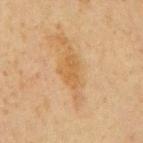No biopsy was performed on this lesion — it was imaged during a full skin examination and was not determined to be concerning.
The subject is a male roughly 60 years of age.
From the mid back.
Captured under cross-polarized illumination.
A close-up tile cropped from a whole-body skin photograph, about 15 mm across.
Approximately 3.5 mm at its widest.